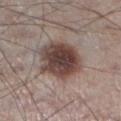follow-up: catalogued during a skin exam; not biopsied | tile lighting: white-light illumination | size: ~6.5 mm (longest diameter) | anatomic site: the left lower leg | image-analysis metrics: a lesion area of about 21 mm², an outline eccentricity of about 0.75 (0 = round, 1 = elongated), and a shape-asymmetry score of about 0.15 (0 = symmetric); a border-irregularity rating of about 2/10 and a color-variation rating of about 5.5/10 | acquisition: ~15 mm crop, total-body skin-cancer survey | subject: male, approximately 60 years of age.